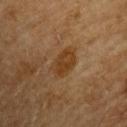Longest diameter approximately 3.5 mm. Captured under cross-polarized illumination. The lesion is located on the chest. A male subject roughly 65 years of age. A roughly 15 mm field-of-view crop from a total-body skin photograph.